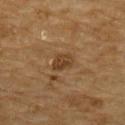Imaged during a routine full-body skin examination; the lesion was not biopsied and no histopathology is available. This is a cross-polarized tile. On the upper back. A 15 mm crop from a total-body photograph taken for skin-cancer surveillance. The patient is a male aged 83 or over.